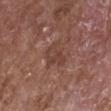follow-up: no biopsy performed (imaged during a skin exam)
diameter: ≈3.5 mm
subject: male, aged approximately 65
image-analysis metrics: a mean CIELAB color near L≈41 a*≈21 b*≈24 and a normalized lesion–skin contrast near 5.5; a border-irregularity index near 3.5/10, a color-variation rating of about 3.5/10, and radial color variation of about 1
image source: ~15 mm crop, total-body skin-cancer survey
illumination: white-light illumination
body site: the right forearm